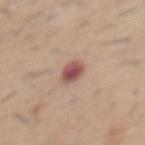Findings:
– notes: no biopsy performed (imaged during a skin exam)
– illumination: white-light illumination
– subject: male, roughly 60 years of age
– anatomic site: the abdomen
– acquisition: total-body-photography crop, ~15 mm field of view